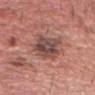Clinical impression:
Imaged during a routine full-body skin examination; the lesion was not biopsied and no histopathology is available.
Context:
An algorithmic analysis of the crop reported border irregularity of about 3.5 on a 0–10 scale, a within-lesion color-variation index near 7/10, and radial color variation of about 2. It also reported an automated nevus-likeness rating near 0 out of 100. Captured under white-light illumination. The patient is a male aged 68–72. A 15 mm close-up extracted from a 3D total-body photography capture. Located on the head or neck. The lesion's longest dimension is about 5.5 mm.